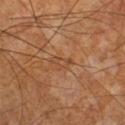Clinical impression:
The lesion was photographed on a routine skin check and not biopsied; there is no pathology result.
Image and clinical context:
The subject is a male aged around 60. Cropped from a total-body skin-imaging series; the visible field is about 15 mm. The lesion is on the right lower leg.